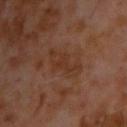Impression: The lesion was tiled from a total-body skin photograph and was not biopsied. Acquisition and patient details: The lesion-visualizer software estimated a footprint of about 8.5 mm². The lesion's longest dimension is about 5.5 mm. On the chest. A region of skin cropped from a whole-body photographic capture, roughly 15 mm wide. A male patient, aged 58–62. Captured under cross-polarized illumination.